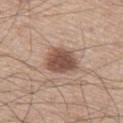biopsy status — catalogued during a skin exam; not biopsied | imaging modality — ~15 mm tile from a whole-body skin photo | lesion size — about 4 mm | TBP lesion metrics — an area of roughly 11 mm², an eccentricity of roughly 0.6, and a symmetry-axis asymmetry near 0.25; a detector confidence of about 100 out of 100 that the crop contains a lesion | tile lighting — white-light | patient — male, about 60 years old | anatomic site — the left thigh.